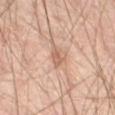Q: Is there a histopathology result?
A: catalogued during a skin exam; not biopsied
Q: Illumination type?
A: cross-polarized
Q: What did automated image analysis measure?
A: an average lesion color of about L≈59 a*≈20 b*≈29 (CIELAB), a lesion–skin lightness drop of about 9, and a normalized lesion–skin contrast near 6.5; a border-irregularity index near 3.5/10
Q: How was this image acquired?
A: 15 mm crop, total-body photography
Q: Who is the patient?
A: male, aged around 65
Q: Where on the body is the lesion?
A: the front of the torso